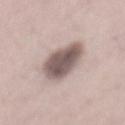Case summary:
* biopsy status — catalogued during a skin exam; not biopsied
* patient — male, in their 70s
* image source — ~15 mm tile from a whole-body skin photo
* illumination — white-light
* site — the back